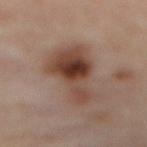This lesion was catalogued during total-body skin photography and was not selected for biopsy. Captured under cross-polarized illumination. This image is a 15 mm lesion crop taken from a total-body photograph. The lesion is located on the mid back. A female patient aged approximately 60. Automated tile analysis of the lesion measured an eccentricity of roughly 0.9 and a symmetry-axis asymmetry near 0.5. And it measured a border-irregularity rating of about 5.5/10, a within-lesion color-variation index near 8.5/10, and radial color variation of about 2.5. The software also gave a detector confidence of about 100 out of 100 that the crop contains a lesion. Approximately 8 mm at its widest.